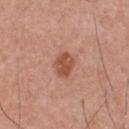{
  "biopsy_status": "not biopsied; imaged during a skin examination",
  "patient": {
    "sex": "male",
    "age_approx": 55
  },
  "lesion_size": {
    "long_diameter_mm_approx": 3.0
  },
  "image": {
    "source": "total-body photography crop",
    "field_of_view_mm": 15
  },
  "site": "chest",
  "automated_metrics": {
    "area_mm2_approx": 6.0,
    "eccentricity": 0.6,
    "shape_asymmetry": 0.2,
    "vs_skin_darker_L": 11.0,
    "vs_skin_contrast_norm": 8.0,
    "border_irregularity_0_10": 2.0,
    "color_variation_0_10": 2.5
  }
}